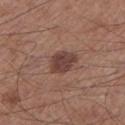Case summary:
– workup: total-body-photography surveillance lesion; no biopsy
– subject: male, aged 58 to 62
– body site: the left lower leg
– image: total-body-photography crop, ~15 mm field of view